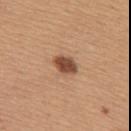Background: Located on the upper back. Cropped from a whole-body photographic skin survey; the tile spans about 15 mm. A female patient, about 50 years old.